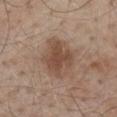Q: Is there a histopathology result?
A: total-body-photography surveillance lesion; no biopsy
Q: What kind of image is this?
A: total-body-photography crop, ~15 mm field of view
Q: Where on the body is the lesion?
A: the mid back
Q: Patient demographics?
A: male, aged 53–57